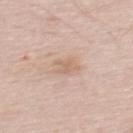biopsy status — catalogued during a skin exam; not biopsied
imaging modality — total-body-photography crop, ~15 mm field of view
illumination — white-light
TBP lesion metrics — border irregularity of about 4 on a 0–10 scale and radial color variation of about 0.5
lesion size — about 2.5 mm
body site — the mid back
patient — male, approximately 55 years of age